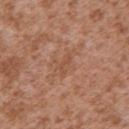Findings:
* notes — total-body-photography surveillance lesion; no biopsy
* site — the left upper arm
* lighting — white-light illumination
* image — ~15 mm crop, total-body skin-cancer survey
* diameter — about 3 mm
* image-analysis metrics — an area of roughly 3.5 mm², a shape eccentricity near 0.85, and a shape-asymmetry score of about 0.5 (0 = symmetric); a mean CIELAB color near L≈51 a*≈22 b*≈32 and roughly 6 lightness units darker than nearby skin; border irregularity of about 5 on a 0–10 scale, a within-lesion color-variation index near 1/10, and radial color variation of about 0.5; a classifier nevus-likeness of about 0/100
* subject — male, aged 43 to 47